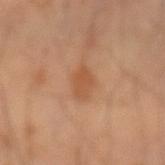A male patient, aged around 65. Located on the right forearm. A 15 mm crop from a total-body photograph taken for skin-cancer surveillance.